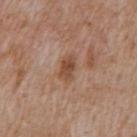Notes:
* imaging modality · total-body-photography crop, ~15 mm field of view
* diameter · ~3 mm (longest diameter)
* location · the mid back
* tile lighting · white-light
* automated metrics · a classifier nevus-likeness of about 50/100 and a detector confidence of about 100 out of 100 that the crop contains a lesion
* patient · male, about 60 years old